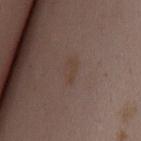The lesion was photographed on a routine skin check and not biopsied; there is no pathology result.
A female subject, roughly 35 years of age.
From the mid back.
A 15 mm close-up tile from a total-body photography series done for melanoma screening.
The recorded lesion diameter is about 3 mm.